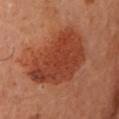Notes:
– follow-up — catalogued during a skin exam; not biopsied
– image — ~15 mm tile from a whole-body skin photo
– lighting — cross-polarized illumination
– lesion size — ≈7.5 mm
– TBP lesion metrics — a lesion area of about 35 mm², an eccentricity of roughly 0.55, and a shape-asymmetry score of about 0.3 (0 = symmetric); a detector confidence of about 100 out of 100 that the crop contains a lesion
– site — the left arm
– patient — female, roughly 60 years of age